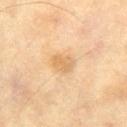Assessment: Imaged during a routine full-body skin examination; the lesion was not biopsied and no histopathology is available. Clinical summary: An algorithmic analysis of the crop reported border irregularity of about 2.5 on a 0–10 scale. The lesion is located on the right thigh. A 15 mm close-up tile from a total-body photography series done for melanoma screening. Approximately 3 mm at its widest. The patient is a male in their 70s.